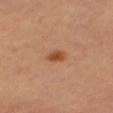Assessment: This lesion was catalogued during total-body skin photography and was not selected for biopsy. Acquisition and patient details: The subject is a female aged approximately 60. The total-body-photography lesion software estimated an eccentricity of roughly 0.8. The analysis additionally found an average lesion color of about L≈50 a*≈26 b*≈37 (CIELAB), roughly 11 lightness units darker than nearby skin, and a normalized border contrast of about 8.5. The analysis additionally found a border-irregularity rating of about 1.5/10, a within-lesion color-variation index near 3.5/10, and a peripheral color-asymmetry measure near 1. The software also gave a classifier nevus-likeness of about 100/100 and a detector confidence of about 100 out of 100 that the crop contains a lesion. A close-up tile cropped from a whole-body skin photograph, about 15 mm across. Located on the right thigh. Measured at roughly 2.5 mm in maximum diameter. Imaged with cross-polarized lighting.